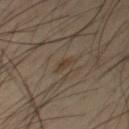| feature | finding |
|---|---|
| follow-up | no biopsy performed (imaged during a skin exam) |
| automated metrics | an area of roughly 2.5 mm², an outline eccentricity of about 0.9 (0 = round, 1 = elongated), and a shape-asymmetry score of about 0.3 (0 = symmetric); a mean CIELAB color near L≈37 a*≈12 b*≈24; a lesion-detection confidence of about 90/100 |
| patient | male, aged approximately 60 |
| image source | ~15 mm tile from a whole-body skin photo |
| lesion diameter | ~2.5 mm (longest diameter) |
| site | the chest |
| tile lighting | cross-polarized |